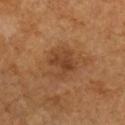{"biopsy_status": "not biopsied; imaged during a skin examination", "lighting": "cross-polarized", "image": {"source": "total-body photography crop", "field_of_view_mm": 15}, "lesion_size": {"long_diameter_mm_approx": 3.5}, "automated_metrics": {"area_mm2_approx": 10.0, "eccentricity": 0.5, "cielab_L": 39, "cielab_a": 20, "cielab_b": 32, "vs_skin_darker_L": 7.0, "vs_skin_contrast_norm": 6.5, "nevus_likeness_0_100": 10, "lesion_detection_confidence_0_100": 100}, "site": "right forearm", "patient": {"sex": "female", "age_approx": 60}}